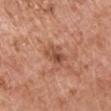Assessment:
Part of a total-body skin-imaging series; this lesion was reviewed on a skin check and was not flagged for biopsy.
Background:
A close-up tile cropped from a whole-body skin photograph, about 15 mm across. The lesion is on the left upper arm. A male patient aged approximately 75.